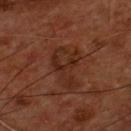Background: This is a cross-polarized tile. A close-up tile cropped from a whole-body skin photograph, about 15 mm across. The recorded lesion diameter is about 5 mm. On the chest. A male patient, aged around 55.